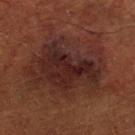Clinical impression:
Part of a total-body skin-imaging series; this lesion was reviewed on a skin check and was not flagged for biopsy.
Image and clinical context:
A region of skin cropped from a whole-body photographic capture, roughly 15 mm wide. The subject is a female aged around 80. From the left lower leg. The tile uses cross-polarized illumination. The lesion's longest dimension is about 11 mm.